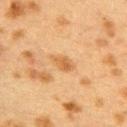{
  "biopsy_status": "not biopsied; imaged during a skin examination",
  "image": {
    "source": "total-body photography crop",
    "field_of_view_mm": 15
  },
  "patient": {
    "sex": "female",
    "age_approx": 40
  },
  "lesion_size": {
    "long_diameter_mm_approx": 3.0
  },
  "automated_metrics": {
    "area_mm2_approx": 4.0,
    "eccentricity": 0.85,
    "shape_asymmetry": 0.25,
    "color_variation_0_10": 2.0,
    "peripheral_color_asymmetry": 0.5,
    "nevus_likeness_0_100": 5
  },
  "site": "upper back"
}